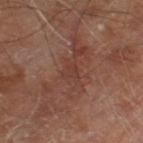Impression:
Part of a total-body skin-imaging series; this lesion was reviewed on a skin check and was not flagged for biopsy.
Background:
The lesion's longest dimension is about 2.5 mm. Captured under cross-polarized illumination. From the left thigh. A male subject aged 68 to 72. An algorithmic analysis of the crop reported a border-irregularity rating of about 4/10, internal color variation of about 1 on a 0–10 scale, and peripheral color asymmetry of about 0.5. And it measured a classifier nevus-likeness of about 0/100 and lesion-presence confidence of about 85/100. Cropped from a total-body skin-imaging series; the visible field is about 15 mm.